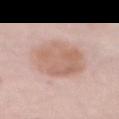{"biopsy_status": "not biopsied; imaged during a skin examination", "patient": {"sex": "female", "age_approx": 70}, "lesion_size": {"long_diameter_mm_approx": 6.5}, "automated_metrics": {"area_mm2_approx": 23.0, "eccentricity": 0.75, "shape_asymmetry": 0.1, "nevus_likeness_0_100": 10, "lesion_detection_confidence_0_100": 100}, "image": {"source": "total-body photography crop", "field_of_view_mm": 15}, "lighting": "white-light", "site": "abdomen"}